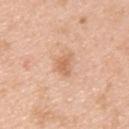This lesion was catalogued during total-body skin photography and was not selected for biopsy.
Located on the back.
The subject is a male aged 33 to 37.
A roughly 15 mm field-of-view crop from a total-body skin photograph.
Approximately 3 mm at its widest.
An algorithmic analysis of the crop reported a footprint of about 4.5 mm² and a shape-asymmetry score of about 0.35 (0 = symmetric). It also reported an average lesion color of about L≈65 a*≈22 b*≈35 (CIELAB), about 9 CIELAB-L* units darker than the surrounding skin, and a normalized border contrast of about 6. And it measured a classifier nevus-likeness of about 5/100.